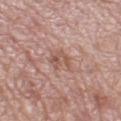biopsy status=catalogued during a skin exam; not biopsied | location=the left thigh | lesion diameter=about 3 mm | tile lighting=white-light illumination | subject=female, aged 48–52 | imaging modality=~15 mm tile from a whole-body skin photo.